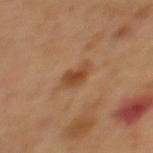Notes:
– follow-up — total-body-photography surveillance lesion; no biopsy
– acquisition — ~15 mm crop, total-body skin-cancer survey
– location — the mid back
– tile lighting — cross-polarized illumination
– patient — male, aged 63–67